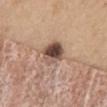biopsy status = no biopsy performed (imaged during a skin exam) | body site = the mid back | lesion diameter = about 3.5 mm | patient = female, in their mid-40s | tile lighting = white-light illumination | acquisition = total-body-photography crop, ~15 mm field of view.